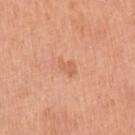Impression:
Captured during whole-body skin photography for melanoma surveillance; the lesion was not biopsied.
Image and clinical context:
A close-up tile cropped from a whole-body skin photograph, about 15 mm across. A female patient, aged approximately 60. From the left upper arm. The recorded lesion diameter is about 2.5 mm. An algorithmic analysis of the crop reported about 7 CIELAB-L* units darker than the surrounding skin. It also reported a border-irregularity index near 4/10 and internal color variation of about 1 on a 0–10 scale. The software also gave a nevus-likeness score of about 5/100. Captured under white-light illumination.